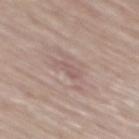<record>
  <biopsy_status>not biopsied; imaged during a skin examination</biopsy_status>
  <patient>
    <sex>male</sex>
    <age_approx>80</age_approx>
  </patient>
  <lighting>white-light</lighting>
  <site>back</site>
  <image>
    <source>total-body photography crop</source>
    <field_of_view_mm>15</field_of_view_mm>
  </image>
</record>